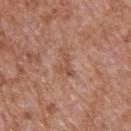biopsy status=catalogued during a skin exam; not biopsied
body site=the upper back
acquisition=15 mm crop, total-body photography
patient=male, aged 63–67
lesion size=~3.5 mm (longest diameter)
automated lesion analysis=border irregularity of about 6 on a 0–10 scale